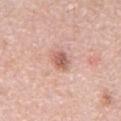No biopsy was performed on this lesion — it was imaged during a full skin examination and was not determined to be concerning. The patient is a male aged approximately 60. The lesion is located on the abdomen. A 15 mm close-up tile from a total-body photography series done for melanoma screening.